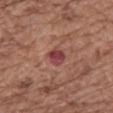The tile uses white-light illumination. This image is a 15 mm lesion crop taken from a total-body photograph. The lesion is located on the mid back. A female subject, aged around 75.